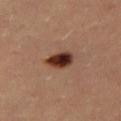workup: no biopsy performed (imaged during a skin exam); body site: the right thigh; lighting: cross-polarized; acquisition: ~15 mm tile from a whole-body skin photo; patient: female, aged approximately 30; lesion diameter: about 3.5 mm.